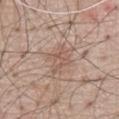Case summary:
• biopsy status: no biopsy performed (imaged during a skin exam)
• acquisition: ~15 mm tile from a whole-body skin photo
• TBP lesion metrics: a footprint of about 6.5 mm² and an outline eccentricity of about 0.7 (0 = round, 1 = elongated); a border-irregularity rating of about 4/10 and radial color variation of about 1; a nevus-likeness score of about 0/100 and a lesion-detection confidence of about 95/100
• lighting: white-light
• body site: the chest
• lesion size: ~3.5 mm (longest diameter)
• patient: male, roughly 70 years of age